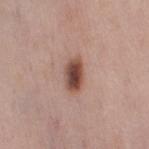| feature | finding |
|---|---|
| biopsy status | imaged on a skin check; not biopsied |
| automated lesion analysis | an area of roughly 6.5 mm², an outline eccentricity of about 0.85 (0 = round, 1 = elongated), and a symmetry-axis asymmetry near 0.1; a lesion color around L≈47 a*≈21 b*≈25 in CIELAB, roughly 17 lightness units darker than nearby skin, and a normalized border contrast of about 11.5; border irregularity of about 1.5 on a 0–10 scale, internal color variation of about 5.5 on a 0–10 scale, and a peripheral color-asymmetry measure near 1.5 |
| acquisition | ~15 mm tile from a whole-body skin photo |
| diameter | ~3.5 mm (longest diameter) |
| illumination | white-light illumination |
| patient | female, aged 48–52 |
| body site | the right thigh |